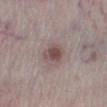biopsy_status: not biopsied; imaged during a skin examination
automated_metrics:
  vs_skin_contrast_norm: 8.5
  nevus_likeness_0_100: 65
  lesion_detection_confidence_0_100: 100
image:
  source: total-body photography crop
  field_of_view_mm: 15
lighting: white-light
patient:
  sex: male
  age_approx: 75
lesion_size:
  long_diameter_mm_approx: 2.5
site: leg